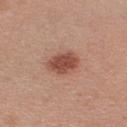<record>
<biopsy_status>not biopsied; imaged during a skin examination</biopsy_status>
<patient>
  <sex>female</sex>
  <age_approx>40</age_approx>
</patient>
<lighting>white-light</lighting>
<site>arm</site>
<lesion_size>
  <long_diameter_mm_approx>4.0</long_diameter_mm_approx>
</lesion_size>
<image>
  <source>total-body photography crop</source>
  <field_of_view_mm>15</field_of_view_mm>
</image>
</record>